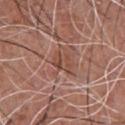From the chest.
The lesion-visualizer software estimated a mean CIELAB color near L≈47 a*≈23 b*≈27. The analysis additionally found a classifier nevus-likeness of about 0/100.
A male subject, in their mid- to late 50s.
A 15 mm crop from a total-body photograph taken for skin-cancer surveillance.